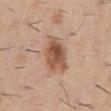Assessment:
Part of a total-body skin-imaging series; this lesion was reviewed on a skin check and was not flagged for biopsy.
Background:
From the abdomen. A male patient about 30 years old. The tile uses white-light illumination. A close-up tile cropped from a whole-body skin photograph, about 15 mm across. Automated tile analysis of the lesion measured a border-irregularity index near 3/10. The software also gave a lesion-detection confidence of about 100/100.